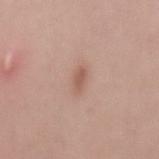workup: total-body-photography surveillance lesion; no biopsy
lighting: white-light illumination
subject: female, in their mid- to late 20s
image source: 15 mm crop, total-body photography
location: the mid back